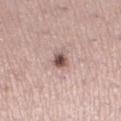{"biopsy_status": "not biopsied; imaged during a skin examination", "patient": {"sex": "female", "age_approx": 40}, "site": "left lower leg", "image": {"source": "total-body photography crop", "field_of_view_mm": 15}, "automated_metrics": {"area_mm2_approx": 3.5, "eccentricity": 0.6, "shape_asymmetry": 0.2, "cielab_L": 52, "cielab_a": 18, "cielab_b": 20, "vs_skin_darker_L": 16.0, "vs_skin_contrast_norm": 10.5, "border_irregularity_0_10": 2.0, "color_variation_0_10": 3.5, "peripheral_color_asymmetry": 1.0, "nevus_likeness_0_100": 85, "lesion_detection_confidence_0_100": 100}}